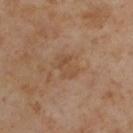Impression: The lesion was photographed on a routine skin check and not biopsied; there is no pathology result. Context: The patient is a male aged 53 to 57. The lesion's longest dimension is about 3 mm. Imaged with cross-polarized lighting. From the upper back. A region of skin cropped from a whole-body photographic capture, roughly 15 mm wide. Automated image analysis of the tile measured a shape-asymmetry score of about 0.25 (0 = symmetric). The software also gave an average lesion color of about L≈49 a*≈17 b*≈32 (CIELAB), about 6 CIELAB-L* units darker than the surrounding skin, and a normalized lesion–skin contrast near 5.